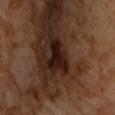workup: imaged on a skin check; not biopsied | automated lesion analysis: a footprint of about 7.5 mm² and two-axis asymmetry of about 0.6; a classifier nevus-likeness of about 0/100 and a lesion-detection confidence of about 95/100 | image: total-body-photography crop, ~15 mm field of view | illumination: cross-polarized | subject: male, about 65 years old | lesion diameter: ~4 mm (longest diameter).